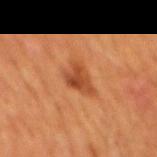The lesion was tiled from a total-body skin photograph and was not biopsied. A male patient, in their mid-60s. The lesion is located on the mid back. Longest diameter approximately 3.5 mm. A 15 mm close-up extracted from a 3D total-body photography capture. Captured under cross-polarized illumination.